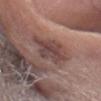The lesion was photographed on a routine skin check and not biopsied; there is no pathology result.
Imaged with white-light lighting.
A 15 mm close-up tile from a total-body photography series done for melanoma screening.
A male subject approximately 75 years of age.
From the head or neck.